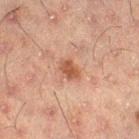The lesion was tiled from a total-body skin photograph and was not biopsied. This image is a 15 mm lesion crop taken from a total-body photograph. A male patient approximately 50 years of age. On the leg. The recorded lesion diameter is about 2.5 mm.